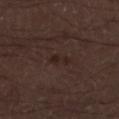Captured during whole-body skin photography for melanoma surveillance; the lesion was not biopsied. A male patient, aged 28–32. Cropped from a total-body skin-imaging series; the visible field is about 15 mm. Imaged with white-light lighting. Automated tile analysis of the lesion measured about 5 CIELAB-L* units darker than the surrounding skin and a normalized border contrast of about 6. The software also gave a border-irregularity index near 4/10, a color-variation rating of about 1/10, and radial color variation of about 0. The software also gave a classifier nevus-likeness of about 5/100 and a detector confidence of about 100 out of 100 that the crop contains a lesion. The lesion's longest dimension is about 2.5 mm. The lesion is on the left lower leg.